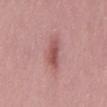A male patient aged 28–32.
The lesion's longest dimension is about 4.5 mm.
The lesion is on the mid back.
Cropped from a whole-body photographic skin survey; the tile spans about 15 mm.